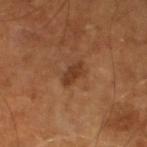<tbp_lesion>
  <biopsy_status>not biopsied; imaged during a skin examination</biopsy_status>
  <automated_metrics>
    <area_mm2_approx>4.0</area_mm2_approx>
    <eccentricity>0.85</eccentricity>
    <shape_asymmetry>0.3</shape_asymmetry>
    <border_irregularity_0_10>3.0</border_irregularity_0_10>
    <color_variation_0_10>1.5</color_variation_0_10>
    <peripheral_color_asymmetry>0.5</peripheral_color_asymmetry>
  </automated_metrics>
  <patient>
    <sex>male</sex>
    <age_approx>65</age_approx>
  </patient>
  <lesion_size>
    <long_diameter_mm_approx>3.0</long_diameter_mm_approx>
  </lesion_size>
  <lighting>cross-polarized</lighting>
  <image>
    <source>total-body photography crop</source>
    <field_of_view_mm>15</field_of_view_mm>
  </image>
</tbp_lesion>